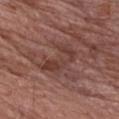Captured during whole-body skin photography for melanoma surveillance; the lesion was not biopsied. The lesion is located on the chest. A 15 mm crop from a total-body photograph taken for skin-cancer surveillance. A male subject, in their 70s.